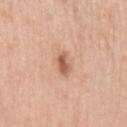<case>
  <biopsy_status>not biopsied; imaged during a skin examination</biopsy_status>
  <site>left upper arm</site>
  <lighting>white-light</lighting>
  <image>
    <source>total-body photography crop</source>
    <field_of_view_mm>15</field_of_view_mm>
  </image>
  <lesion_size>
    <long_diameter_mm_approx>2.5</long_diameter_mm_approx>
  </lesion_size>
  <patient>
    <sex>male</sex>
    <age_approx>55</age_approx>
  </patient>
</case>